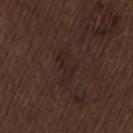No biopsy was performed on this lesion — it was imaged during a full skin examination and was not determined to be concerning. A male patient, aged 68–72. The lesion is located on the back. A lesion tile, about 15 mm wide, cut from a 3D total-body photograph. Captured under white-light illumination.